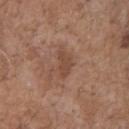{
  "biopsy_status": "not biopsied; imaged during a skin examination",
  "lighting": "white-light",
  "patient": {
    "sex": "male",
    "age_approx": 70
  },
  "site": "chest",
  "image": {
    "source": "total-body photography crop",
    "field_of_view_mm": 15
  }
}